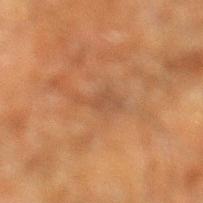follow-up: total-body-photography surveillance lesion; no biopsy
tile lighting: cross-polarized illumination
subject: male, roughly 60 years of age
acquisition: ~15 mm crop, total-body skin-cancer survey
size: about 4 mm
anatomic site: the left lower leg